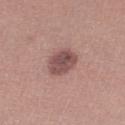Captured during whole-body skin photography for melanoma surveillance; the lesion was not biopsied. A female subject, in their mid-30s. Approximately 3.5 mm at its widest. On the left leg. This is a white-light tile. A lesion tile, about 15 mm wide, cut from a 3D total-body photograph.